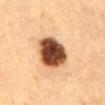Assessment: The lesion was tiled from a total-body skin photograph and was not biopsied. Image and clinical context: A lesion tile, about 15 mm wide, cut from a 3D total-body photograph. A female patient aged approximately 60. The lesion is on the abdomen. The tile uses cross-polarized illumination. The total-body-photography lesion software estimated an eccentricity of roughly 0.35. It also reported an average lesion color of about L≈39 a*≈19 b*≈29 (CIELAB), roughly 23 lightness units darker than nearby skin, and a normalized lesion–skin contrast near 16.5. The software also gave peripheral color asymmetry of about 2. Approximately 4.5 mm at its widest.